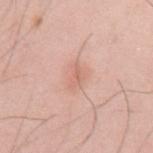Assessment:
Part of a total-body skin-imaging series; this lesion was reviewed on a skin check and was not flagged for biopsy.
Background:
Cropped from a total-body skin-imaging series; the visible field is about 15 mm. Measured at roughly 3 mm in maximum diameter. A male subject, aged approximately 30. The lesion is on the left upper arm. Imaged with white-light lighting.